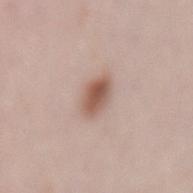| key | value |
|---|---|
| biopsy status | catalogued during a skin exam; not biopsied |
| illumination | white-light illumination |
| diameter | ≈3.5 mm |
| acquisition | ~15 mm crop, total-body skin-cancer survey |
| subject | female, aged approximately 65 |
| anatomic site | the mid back |